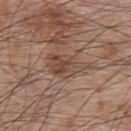Q: Is there a histopathology result?
A: no biopsy performed (imaged during a skin exam)
Q: What lighting was used for the tile?
A: white-light illumination
Q: Who is the patient?
A: male, aged around 65
Q: What is the imaging modality?
A: total-body-photography crop, ~15 mm field of view
Q: Automated lesion metrics?
A: a lesion area of about 9.5 mm², an eccentricity of roughly 0.85, and two-axis asymmetry of about 0.35; a within-lesion color-variation index near 5.5/10; an automated nevus-likeness rating near 5 out of 100 and a lesion-detection confidence of about 95/100
Q: What is the anatomic site?
A: the back
Q: How large is the lesion?
A: ~5.5 mm (longest diameter)